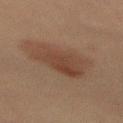biopsy status=catalogued during a skin exam; not biopsied
subject=male, aged approximately 60
diameter=about 6.5 mm
body site=the mid back
image source=~15 mm crop, total-body skin-cancer survey
lighting=cross-polarized